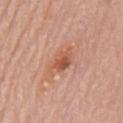  biopsy_status: not biopsied; imaged during a skin examination
  patient:
    sex: male
    age_approx: 80
  site: chest
  image:
    source: total-body photography crop
    field_of_view_mm: 15